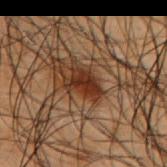Recorded during total-body skin imaging; not selected for excision or biopsy. Automated tile analysis of the lesion measured a color-variation rating of about 3/10 and a peripheral color-asymmetry measure near 1. The patient is a male in their 50s. Located on the right upper arm. Cropped from a total-body skin-imaging series; the visible field is about 15 mm.